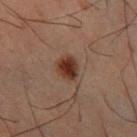This lesion was catalogued during total-body skin photography and was not selected for biopsy.